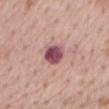Impression:
Imaged during a routine full-body skin examination; the lesion was not biopsied and no histopathology is available.
Acquisition and patient details:
The subject is a female roughly 65 years of age. An algorithmic analysis of the crop reported an area of roughly 7 mm², a shape eccentricity near 0.5, and two-axis asymmetry of about 0.2. It also reported an average lesion color of about L≈50 a*≈27 b*≈16 (CIELAB), roughly 17 lightness units darker than nearby skin, and a normalized lesion–skin contrast near 12.5. The lesion is located on the mid back. A 15 mm crop from a total-body photograph taken for skin-cancer surveillance. Approximately 3 mm at its widest. The tile uses white-light illumination.